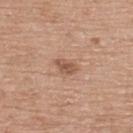Part of a total-body skin-imaging series; this lesion was reviewed on a skin check and was not flagged for biopsy.
This is a white-light tile.
This image is a 15 mm lesion crop taken from a total-body photograph.
On the upper back.
The recorded lesion diameter is about 2.5 mm.
A female subject aged 58 to 62.